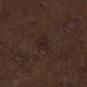• workup: total-body-photography surveillance lesion; no biopsy
• illumination: white-light
• subject: male, aged approximately 70
• automated metrics: a classifier nevus-likeness of about 0/100 and a lesion-detection confidence of about 100/100
• acquisition: total-body-photography crop, ~15 mm field of view
• site: the mid back